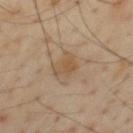No biopsy was performed on this lesion — it was imaged during a full skin examination and was not determined to be concerning.